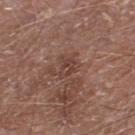location: the right lower leg | image: total-body-photography crop, ~15 mm field of view | lesion size: ≈2.5 mm | subject: male, aged 68–72 | illumination: white-light illumination.